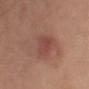follow-up: total-body-photography surveillance lesion; no biopsy | acquisition: 15 mm crop, total-body photography | subject: male, approximately 70 years of age | illumination: white-light | site: the arm.